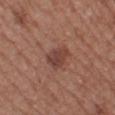biopsy status: no biopsy performed (imaged during a skin exam) | subject: female, aged around 40 | size: ≈3.5 mm | location: the left thigh | automated lesion analysis: a lesion color around L≈42 a*≈23 b*≈25 in CIELAB, about 9 CIELAB-L* units darker than the surrounding skin, and a normalized border contrast of about 7.5; a border-irregularity index near 3/10, a color-variation rating of about 3/10, and radial color variation of about 1; a classifier nevus-likeness of about 75/100 | image: ~15 mm crop, total-body skin-cancer survey.